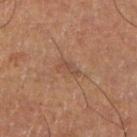The lesion was photographed on a routine skin check and not biopsied; there is no pathology result. Captured under cross-polarized illumination. A 15 mm crop from a total-body photograph taken for skin-cancer surveillance. Located on the right lower leg. A male patient aged around 55. About 3 mm across.